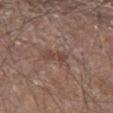follow-up — imaged on a skin check; not biopsied | location — the left upper arm | automated metrics — an area of roughly 3.5 mm², a shape eccentricity near 0.85, and two-axis asymmetry of about 0.4; border irregularity of about 5 on a 0–10 scale and radial color variation of about 1; a classifier nevus-likeness of about 0/100 | patient — male, about 85 years old or older | size — about 3 mm | image source — total-body-photography crop, ~15 mm field of view | illumination — white-light illumination.